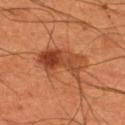An algorithmic analysis of the crop reported an outline eccentricity of about 0.75 (0 = round, 1 = elongated) and a shape-asymmetry score of about 0.35 (0 = symmetric). And it measured a classifier nevus-likeness of about 85/100 and a detector confidence of about 100 out of 100 that the crop contains a lesion. The recorded lesion diameter is about 6 mm. A region of skin cropped from a whole-body photographic capture, roughly 15 mm wide. This is a cross-polarized tile. The lesion is on the left thigh. A male patient, aged approximately 55.